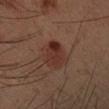| feature | finding |
|---|---|
| location | the left forearm |
| lighting | cross-polarized |
| subject | male, aged 48–52 |
| acquisition | ~15 mm crop, total-body skin-cancer survey |
| image-analysis metrics | a mean CIELAB color near L≈27 a*≈19 b*≈22 and a lesion-to-skin contrast of about 8 (normalized; higher = more distinct); a nevus-likeness score of about 60/100 |
| diameter | ≈4 mm |